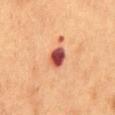Notes:
– automated lesion analysis · a lesion color around L≈46 a*≈31 b*≈28 in CIELAB, a lesion–skin lightness drop of about 19, and a normalized border contrast of about 13.5; a border-irregularity rating of about 2/10, internal color variation of about 8 on a 0–10 scale, and radial color variation of about 2.5
– diameter · about 3 mm
– body site · the mid back
– patient · male, about 55 years old
– lighting · cross-polarized
– image · 15 mm crop, total-body photography